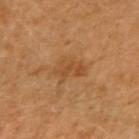Imaged with cross-polarized lighting. This image is a 15 mm lesion crop taken from a total-body photograph. The lesion is located on the right upper arm. A female patient, in their 50s. Longest diameter approximately 3.5 mm.